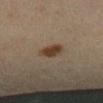  biopsy_status: not biopsied; imaged during a skin examination
  patient:
    sex: female
    age_approx: 45
  image:
    source: total-body photography crop
    field_of_view_mm: 15
  site: leg
  lighting: cross-polarized